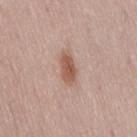| feature | finding |
|---|---|
| size | ≈3.5 mm |
| image | ~15 mm crop, total-body skin-cancer survey |
| site | the lower back |
| subject | female, aged 38–42 |